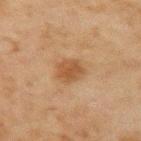Findings:
– workup · no biopsy performed (imaged during a skin exam)
– body site · the left upper arm
– subject · male, aged 43–47
– automated metrics · an area of roughly 6.5 mm² and two-axis asymmetry of about 0.2; an average lesion color of about L≈42 a*≈18 b*≈31 (CIELAB) and about 8 CIELAB-L* units darker than the surrounding skin; a border-irregularity index near 2/10 and a color-variation rating of about 2.5/10; a classifier nevus-likeness of about 65/100 and a detector confidence of about 100 out of 100 that the crop contains a lesion
– image source · ~15 mm tile from a whole-body skin photo
– illumination · cross-polarized illumination